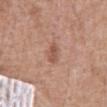- follow-up · total-body-photography surveillance lesion; no biopsy
- subject · male, about 60 years old
- image · ~15 mm crop, total-body skin-cancer survey
- diameter · about 2.5 mm
- anatomic site · the abdomen